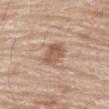No biopsy was performed on this lesion — it was imaged during a full skin examination and was not determined to be concerning. An algorithmic analysis of the crop reported a footprint of about 7 mm², a shape eccentricity near 0.75, and two-axis asymmetry of about 0.25. It also reported a lesion color around L≈57 a*≈19 b*≈30 in CIELAB, roughly 11 lightness units darker than nearby skin, and a normalized lesion–skin contrast near 7.5. The software also gave border irregularity of about 2.5 on a 0–10 scale, a within-lesion color-variation index near 3.5/10, and a peripheral color-asymmetry measure near 1. The lesion is on the abdomen. A male subject in their 80s. A close-up tile cropped from a whole-body skin photograph, about 15 mm across. The lesion's longest dimension is about 3.5 mm. This is a white-light tile.